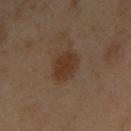<lesion>
  <biopsy_status>not biopsied; imaged during a skin examination</biopsy_status>
  <image>
    <source>total-body photography crop</source>
    <field_of_view_mm>15</field_of_view_mm>
  </image>
  <patient>
    <sex>male</sex>
    <age_approx>55</age_approx>
  </patient>
  <site>arm</site>
  <lesion_size>
    <long_diameter_mm_approx>3.5</long_diameter_mm_approx>
  </lesion_size>
  <lighting>cross-polarized</lighting>
  <automated_metrics>
    <vs_skin_contrast_norm>8.0</vs_skin_contrast_norm>
    <border_irregularity_0_10>2.0</border_irregularity_0_10>
    <color_variation_0_10>1.5</color_variation_0_10>
    <peripheral_color_asymmetry>0.5</peripheral_color_asymmetry>
    <lesion_detection_confidence_0_100>100</lesion_detection_confidence_0_100>
  </automated_metrics>
</lesion>